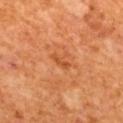<record>
<site>mid back</site>
<lighting>cross-polarized</lighting>
<lesion_size>
  <long_diameter_mm_approx>2.5</long_diameter_mm_approx>
</lesion_size>
<image>
  <source>total-body photography crop</source>
  <field_of_view_mm>15</field_of_view_mm>
</image>
<patient>
  <sex>male</sex>
  <age_approx>65</age_approx>
</patient>
<automated_metrics>
  <area_mm2_approx>3.0</area_mm2_approx>
  <shape_asymmetry>0.2</shape_asymmetry>
</automated_metrics>
</record>